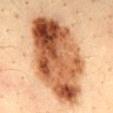Image and clinical context: Approximately 12.5 mm at its widest. Imaged with cross-polarized lighting. A close-up tile cropped from a whole-body skin photograph, about 15 mm across. The total-body-photography lesion software estimated a mean CIELAB color near L≈53 a*≈23 b*≈36, roughly 20 lightness units darker than nearby skin, and a lesion-to-skin contrast of about 12.5 (normalized; higher = more distinct). The subject is a male in their mid- to late 30s. Located on the mid back.